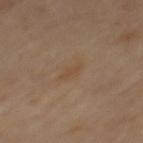notes = total-body-photography surveillance lesion; no biopsy
location = the mid back
imaging modality = total-body-photography crop, ~15 mm field of view
diameter = ~2.5 mm (longest diameter)
tile lighting = cross-polarized